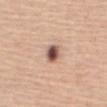{
  "biopsy_status": "not biopsied; imaged during a skin examination",
  "patient": {
    "sex": "female",
    "age_approx": 55
  },
  "lighting": "white-light",
  "site": "front of the torso",
  "image": {
    "source": "total-body photography crop",
    "field_of_view_mm": 15
  },
  "lesion_size": {
    "long_diameter_mm_approx": 2.5
  }
}